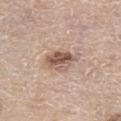Case summary:
• follow-up: total-body-photography surveillance lesion; no biopsy
• location: the left thigh
• image: 15 mm crop, total-body photography
• patient: male, in their 80s